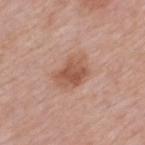Impression: Part of a total-body skin-imaging series; this lesion was reviewed on a skin check and was not flagged for biopsy. Clinical summary: The tile uses white-light illumination. On the back. The subject is a male aged 53 to 57. A close-up tile cropped from a whole-body skin photograph, about 15 mm across.